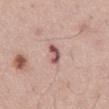{
  "biopsy_status": "not biopsied; imaged during a skin examination",
  "lesion_size": {
    "long_diameter_mm_approx": 3.0
  },
  "automated_metrics": {
    "area_mm2_approx": 4.0,
    "eccentricity": 0.9,
    "shape_asymmetry": 0.35,
    "cielab_L": 55,
    "cielab_a": 23,
    "cielab_b": 21
  },
  "site": "abdomen",
  "image": {
    "source": "total-body photography crop",
    "field_of_view_mm": 15
  },
  "patient": {
    "sex": "male",
    "age_approx": 55
  }
}